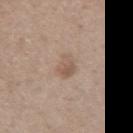| field | value |
|---|---|
| notes | imaged on a skin check; not biopsied |
| lesion size | about 3 mm |
| image | ~15 mm crop, total-body skin-cancer survey |
| lighting | white-light |
| patient | female, about 65 years old |
| anatomic site | the right upper arm |